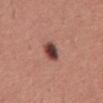Captured during whole-body skin photography for melanoma surveillance; the lesion was not biopsied. A region of skin cropped from a whole-body photographic capture, roughly 15 mm wide. Located on the back. Captured under white-light illumination. A male subject aged 43 to 47.